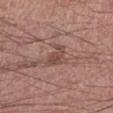– biopsy status: no biopsy performed (imaged during a skin exam)
– site: the right lower leg
– image source: ~15 mm tile from a whole-body skin photo
– size: about 3 mm
– tile lighting: white-light illumination
– automated metrics: a mean CIELAB color near L≈47 a*≈20 b*≈24; a within-lesion color-variation index near 2/10; lesion-presence confidence of about 100/100
– patient: male, in their mid-40s